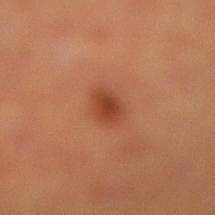• follow-up — catalogued during a skin exam; not biopsied
• lesion size — about 2.5 mm
• site — the leg
• automated metrics — an area of roughly 5 mm², an eccentricity of roughly 0.65, and a shape-asymmetry score of about 0.2 (0 = symmetric); a lesion color around L≈44 a*≈28 b*≈36 in CIELAB, roughly 10 lightness units darker than nearby skin, and a normalized lesion–skin contrast near 8; a border-irregularity index near 1.5/10, a color-variation rating of about 2.5/10, and a peripheral color-asymmetry measure near 0.5
• imaging modality — 15 mm crop, total-body photography
• tile lighting — cross-polarized
• subject — male, aged around 50